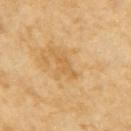The lesion was tiled from a total-body skin photograph and was not biopsied.
An algorithmic analysis of the crop reported a lesion color around L≈64 a*≈20 b*≈46 in CIELAB.
The lesion is on the right upper arm.
A female patient roughly 70 years of age.
A lesion tile, about 15 mm wide, cut from a 3D total-body photograph.
Longest diameter approximately 3.5 mm.